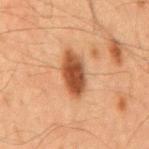Impression: This lesion was catalogued during total-body skin photography and was not selected for biopsy. Image and clinical context: The lesion's longest dimension is about 5.5 mm. Automated image analysis of the tile measured a footprint of about 10 mm². And it measured a border-irregularity index near 2/10, internal color variation of about 4 on a 0–10 scale, and radial color variation of about 1. On the mid back. A lesion tile, about 15 mm wide, cut from a 3D total-body photograph. A male subject, aged 63 to 67.